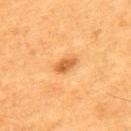• follow-up · catalogued during a skin exam; not biopsied
• location · the back
• TBP lesion metrics · a footprint of about 3.5 mm², an outline eccentricity of about 0.85 (0 = round, 1 = elongated), and two-axis asymmetry of about 0.3; a lesion color around L≈57 a*≈26 b*≈45 in CIELAB
• image source · total-body-photography crop, ~15 mm field of view
• patient · male, aged approximately 60